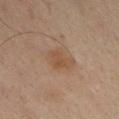  biopsy_status: not biopsied; imaged during a skin examination
  patient:
    sex: male
    age_approx: 50
  site: front of the torso
  lighting: cross-polarized
  image:
    source: total-body photography crop
    field_of_view_mm: 15
  lesion_size:
    long_diameter_mm_approx: 3.5